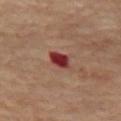  biopsy_status: not biopsied; imaged during a skin examination
  lighting: cross-polarized
  patient:
    sex: female
    age_approx: 65
  image:
    source: total-body photography crop
    field_of_view_mm: 15
  lesion_size:
    long_diameter_mm_approx: 3.0
  site: left thigh
  automated_metrics:
    border_irregularity_0_10: 2.0
    color_variation_0_10: 3.5
    peripheral_color_asymmetry: 1.0